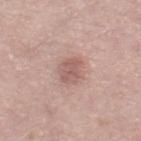<record>
<biopsy_status>not biopsied; imaged during a skin examination</biopsy_status>
<image>
  <source>total-body photography crop</source>
  <field_of_view_mm>15</field_of_view_mm>
</image>
<patient>
  <sex>female</sex>
  <age_approx>65</age_approx>
</patient>
<site>left thigh</site>
</record>